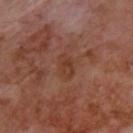– workup: total-body-photography surveillance lesion; no biopsy
– patient: male, aged 68–72
– illumination: cross-polarized
– location: the upper back
– image source: total-body-photography crop, ~15 mm field of view